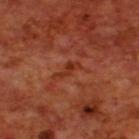{
  "biopsy_status": "not biopsied; imaged during a skin examination",
  "image": {
    "source": "total-body photography crop",
    "field_of_view_mm": 15
  },
  "lesion_size": {
    "long_diameter_mm_approx": 3.0
  },
  "automated_metrics": {
    "border_irregularity_0_10": 5.0,
    "color_variation_0_10": 0.0,
    "peripheral_color_asymmetry": 0.0
  },
  "lighting": "cross-polarized",
  "site": "upper back",
  "patient": {
    "sex": "male",
    "age_approx": 70
  }
}